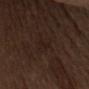* tile lighting · white-light illumination
* anatomic site · the upper back
* image-analysis metrics · a mean CIELAB color near L≈18 a*≈14 b*≈18, a lesion–skin lightness drop of about 3, and a lesion-to-skin contrast of about 5 (normalized; higher = more distinct)
* diameter · ~2.5 mm (longest diameter)
* subject · male, aged around 70
* imaging modality · ~15 mm tile from a whole-body skin photo